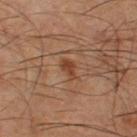Captured during whole-body skin photography for melanoma surveillance; the lesion was not biopsied. Approximately 2.5 mm at its widest. On the right thigh. Captured under cross-polarized illumination. A roughly 15 mm field-of-view crop from a total-body skin photograph. A male subject, aged around 60.